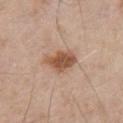Case summary:
- biopsy status: catalogued during a skin exam; not biopsied
- patient: male, in their mid-50s
- automated lesion analysis: a footprint of about 9.5 mm² and a shape eccentricity near 0.75; an average lesion color of about L≈54 a*≈20 b*≈30 (CIELAB), about 12 CIELAB-L* units darker than the surrounding skin, and a lesion-to-skin contrast of about 8.5 (normalized; higher = more distinct); border irregularity of about 3 on a 0–10 scale, a color-variation rating of about 4/10, and radial color variation of about 1
- image source: ~15 mm crop, total-body skin-cancer survey
- size: about 4.5 mm
- illumination: white-light illumination
- body site: the chest